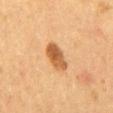The lesion-visualizer software estimated a footprint of about 7.5 mm², an eccentricity of roughly 0.85, and a shape-asymmetry score of about 0.15 (0 = symmetric). The software also gave a lesion color around L≈47 a*≈20 b*≈35 in CIELAB, roughly 12 lightness units darker than nearby skin, and a normalized border contrast of about 9. And it measured a border-irregularity index near 2/10, internal color variation of about 4 on a 0–10 scale, and a peripheral color-asymmetry measure near 1.5.
This is a cross-polarized tile.
About 4 mm across.
Located on the chest.
Cropped from a total-body skin-imaging series; the visible field is about 15 mm.
A female patient, aged around 50.